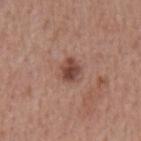The lesion was photographed on a routine skin check and not biopsied; there is no pathology result. A region of skin cropped from a whole-body photographic capture, roughly 15 mm wide. This is a white-light tile. The recorded lesion diameter is about 2.5 mm. On the back. A male subject, in their mid-60s.